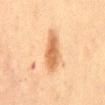Imaged during a routine full-body skin examination; the lesion was not biopsied and no histopathology is available.